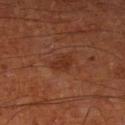| key | value |
|---|---|
| notes | imaged on a skin check; not biopsied |
| lesion diameter | ~3 mm (longest diameter) |
| site | the left lower leg |
| subject | male, aged approximately 65 |
| acquisition | ~15 mm tile from a whole-body skin photo |
| TBP lesion metrics | an eccentricity of roughly 0.7 and a shape-asymmetry score of about 0.3 (0 = symmetric); internal color variation of about 1.5 on a 0–10 scale and peripheral color asymmetry of about 0.5 |